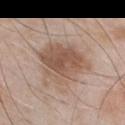biopsy status: total-body-photography surveillance lesion; no biopsy | tile lighting: white-light | body site: the chest | TBP lesion metrics: an average lesion color of about L≈54 a*≈17 b*≈27 (CIELAB) and a normalized border contrast of about 7.5 | imaging modality: total-body-photography crop, ~15 mm field of view | patient: male, about 55 years old.